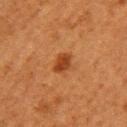  biopsy_status: not biopsied; imaged during a skin examination
  site: left upper arm
  lesion_size:
    long_diameter_mm_approx: 2.5
  patient:
    sex: female
    age_approx: 50
  image:
    source: total-body photography crop
    field_of_view_mm: 15
  automated_metrics:
    eccentricity: 0.65
    border_irregularity_0_10: 1.5
    color_variation_0_10: 2.5
    peripheral_color_asymmetry: 1.0
  lighting: cross-polarized